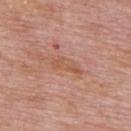Impression: Recorded during total-body skin imaging; not selected for excision or biopsy. Context: A roughly 15 mm field-of-view crop from a total-body skin photograph. Automated image analysis of the tile measured a lesion area of about 5 mm² and two-axis asymmetry of about 0.35. And it measured an average lesion color of about L≈56 a*≈23 b*≈32 (CIELAB) and about 6 CIELAB-L* units darker than the surrounding skin. A male subject approximately 75 years of age. The lesion is on the upper back.